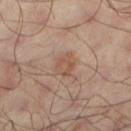subject — male, aged 63–67
acquisition — total-body-photography crop, ~15 mm field of view
diameter — ≈3 mm
TBP lesion metrics — a footprint of about 5.5 mm², a shape eccentricity near 0.5, and a symmetry-axis asymmetry near 0.25; a lesion color around L≈49 a*≈17 b*≈27 in CIELAB, about 7 CIELAB-L* units darker than the surrounding skin, and a lesion-to-skin contrast of about 6 (normalized; higher = more distinct)
site — the left lower leg
lighting — cross-polarized illumination